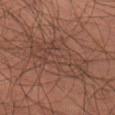Clinical impression:
The lesion was tiled from a total-body skin photograph and was not biopsied.
Acquisition and patient details:
A 15 mm crop from a total-body photograph taken for skin-cancer surveillance. On the left thigh. A male patient aged approximately 45.